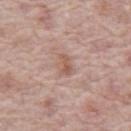Assessment: Recorded during total-body skin imaging; not selected for excision or biopsy. Acquisition and patient details: The subject is a male aged around 70. Automated tile analysis of the lesion measured an eccentricity of roughly 0.75 and a symmetry-axis asymmetry near 0.25. The software also gave a mean CIELAB color near L≈57 a*≈20 b*≈26, a lesion–skin lightness drop of about 8, and a normalized border contrast of about 6. A lesion tile, about 15 mm wide, cut from a 3D total-body photograph. Longest diameter approximately 2.5 mm. On the abdomen.